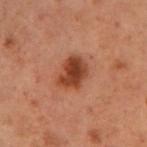Imaged during a routine full-body skin examination; the lesion was not biopsied and no histopathology is available.